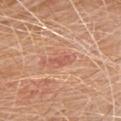A female subject, aged 68–72.
Automated tile analysis of the lesion measured an outline eccentricity of about 0.9 (0 = round, 1 = elongated).
This is a white-light tile.
On the chest.
The recorded lesion diameter is about 3 mm.
A lesion tile, about 15 mm wide, cut from a 3D total-body photograph.